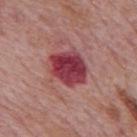- biopsy status · total-body-photography surveillance lesion; no biopsy
- lesion diameter · ~5 mm (longest diameter)
- anatomic site · the mid back
- patient · male, aged 73 to 77
- TBP lesion metrics · an average lesion color of about L≈40 a*≈35 b*≈19 (CIELAB), roughly 16 lightness units darker than nearby skin, and a normalized lesion–skin contrast near 12; a border-irregularity rating of about 1.5/10 and radial color variation of about 1.5
- tile lighting · white-light
- imaging modality · 15 mm crop, total-body photography